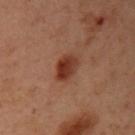Captured during whole-body skin photography for melanoma surveillance; the lesion was not biopsied. Located on the arm. Captured under cross-polarized illumination. A lesion tile, about 15 mm wide, cut from a 3D total-body photograph. A female subject, in their mid-50s.